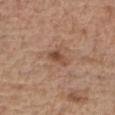Q: Was this lesion biopsied?
A: imaged on a skin check; not biopsied
Q: What did automated image analysis measure?
A: a lesion color around L≈47 a*≈21 b*≈30 in CIELAB and a normalized lesion–skin contrast near 7.5; an automated nevus-likeness rating near 15 out of 100 and a lesion-detection confidence of about 100/100
Q: What are the patient's age and sex?
A: male, approximately 70 years of age
Q: How was this image acquired?
A: ~15 mm tile from a whole-body skin photo
Q: What is the lesion's diameter?
A: about 3 mm
Q: What is the anatomic site?
A: the right forearm